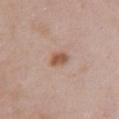{
  "automated_metrics": {
    "eccentricity": 0.7,
    "shape_asymmetry": 0.2,
    "cielab_L": 54,
    "cielab_a": 21,
    "cielab_b": 30,
    "vs_skin_darker_L": 11.0,
    "vs_skin_contrast_norm": 8.5
  },
  "site": "front of the torso",
  "image": {
    "source": "total-body photography crop",
    "field_of_view_mm": 15
  },
  "lesion_size": {
    "long_diameter_mm_approx": 2.5
  },
  "lighting": "white-light",
  "patient": {
    "sex": "female",
    "age_approx": 40
  }
}